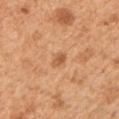Q: Was this lesion biopsied?
A: no biopsy performed (imaged during a skin exam)
Q: What are the patient's age and sex?
A: male, aged around 55
Q: Lesion location?
A: the left upper arm
Q: What kind of image is this?
A: ~15 mm tile from a whole-body skin photo